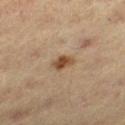Captured during whole-body skin photography for melanoma surveillance; the lesion was not biopsied.